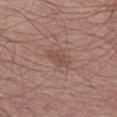biopsy status: imaged on a skin check; not biopsied
image source: 15 mm crop, total-body photography
illumination: white-light
image-analysis metrics: an average lesion color of about L≈49 a*≈19 b*≈24 (CIELAB) and roughly 7 lightness units darker than nearby skin; internal color variation of about 2.5 on a 0–10 scale and a peripheral color-asymmetry measure near 1; a classifier nevus-likeness of about 0/100 and a lesion-detection confidence of about 100/100
lesion diameter: ~3 mm (longest diameter)
patient: male, in their mid- to late 50s
site: the left thigh